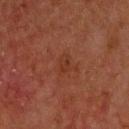<tbp_lesion>
  <biopsy_status>not biopsied; imaged during a skin examination</biopsy_status>
  <automated_metrics>
    <area_mm2_approx>3.0</area_mm2_approx>
    <eccentricity>0.85</eccentricity>
    <shape_asymmetry>0.35</shape_asymmetry>
    <cielab_L>28</cielab_L>
    <cielab_a>21</cielab_a>
    <cielab_b>27</cielab_b>
    <vs_skin_darker_L>4.0</vs_skin_darker_L>
    <vs_skin_contrast_norm>5.0</vs_skin_contrast_norm>
    <border_irregularity_0_10>3.5</border_irregularity_0_10>
    <color_variation_0_10>1.0</color_variation_0_10>
    <peripheral_color_asymmetry>0.5</peripheral_color_asymmetry>
  </automated_metrics>
  <lighting>cross-polarized</lighting>
  <site>upper back</site>
  <lesion_size>
    <long_diameter_mm_approx>2.5</long_diameter_mm_approx>
  </lesion_size>
  <patient>
    <sex>male</sex>
    <age_approx>70</age_approx>
  </patient>
  <image>
    <source>total-body photography crop</source>
    <field_of_view_mm>15</field_of_view_mm>
  </image>
</tbp_lesion>